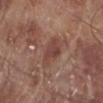Assessment: No biopsy was performed on this lesion — it was imaged during a full skin examination and was not determined to be concerning. Background: A lesion tile, about 15 mm wide, cut from a 3D total-body photograph. From the leg. A male patient, roughly 70 years of age. The tile uses white-light illumination. Automated tile analysis of the lesion measured a lesion area of about 7.5 mm², an outline eccentricity of about 0.75 (0 = round, 1 = elongated), and a symmetry-axis asymmetry near 0.3. The analysis additionally found an average lesion color of about L≈43 a*≈22 b*≈24 (CIELAB), a lesion–skin lightness drop of about 8, and a normalized border contrast of about 6.5. And it measured a classifier nevus-likeness of about 20/100. About 4 mm across.